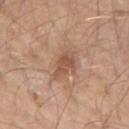notes: imaged on a skin check; not biopsied
automated metrics: roughly 9 lightness units darker than nearby skin and a lesion-to-skin contrast of about 6.5 (normalized; higher = more distinct); a lesion-detection confidence of about 100/100
image: total-body-photography crop, ~15 mm field of view
anatomic site: the left upper arm
diameter: ~4 mm (longest diameter)
patient: male, roughly 60 years of age